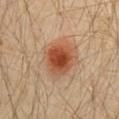Case summary:
- biopsy status: no biopsy performed (imaged during a skin exam)
- body site: the chest
- image: ~15 mm crop, total-body skin-cancer survey
- patient: male, aged 28 to 32